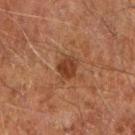The lesion was tiled from a total-body skin photograph and was not biopsied.
The lesion is located on the arm.
A male subject aged 58–62.
A 15 mm close-up extracted from a 3D total-body photography capture.
Imaged with cross-polarized lighting.